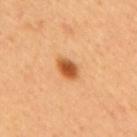The lesion was tiled from a total-body skin photograph and was not biopsied. The patient is a female approximately 45 years of age. The lesion is on the back. The lesion-visualizer software estimated an eccentricity of roughly 0.75 and two-axis asymmetry of about 0.2. The software also gave a border-irregularity index near 2/10 and internal color variation of about 4 on a 0–10 scale. The analysis additionally found a nevus-likeness score of about 100/100 and a detector confidence of about 100 out of 100 that the crop contains a lesion. Approximately 3 mm at its widest. A roughly 15 mm field-of-view crop from a total-body skin photograph.